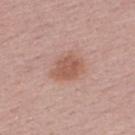| field | value |
|---|---|
| notes | no biopsy performed (imaged during a skin exam) |
| imaging modality | ~15 mm crop, total-body skin-cancer survey |
| subject | male, approximately 30 years of age |
| lighting | white-light |